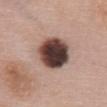Assessment:
Imaged during a routine full-body skin examination; the lesion was not biopsied and no histopathology is available.
Context:
An algorithmic analysis of the crop reported an area of roughly 18 mm², an outline eccentricity of about 0.65 (0 = round, 1 = elongated), and a shape-asymmetry score of about 0.1 (0 = symmetric). The analysis additionally found an average lesion color of about L≈41 a*≈17 b*≈20 (CIELAB), about 25 CIELAB-L* units darker than the surrounding skin, and a normalized border contrast of about 17. It also reported a border-irregularity index near 1/10 and internal color variation of about 9.5 on a 0–10 scale. From the back. Imaged with white-light lighting. This image is a 15 mm lesion crop taken from a total-body photograph. A female subject, roughly 60 years of age.